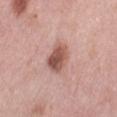Imaged during a routine full-body skin examination; the lesion was not biopsied and no histopathology is available.
Cropped from a total-body skin-imaging series; the visible field is about 15 mm.
About 4 mm across.
Automated tile analysis of the lesion measured an eccentricity of roughly 0.6 and a symmetry-axis asymmetry near 0.2. The analysis additionally found a lesion color around L≈55 a*≈23 b*≈25 in CIELAB, roughly 14 lightness units darker than nearby skin, and a lesion-to-skin contrast of about 9 (normalized; higher = more distinct). The software also gave a border-irregularity index near 1.5/10 and radial color variation of about 2.
A female patient, aged approximately 40.
Imaged with white-light lighting.
On the left thigh.